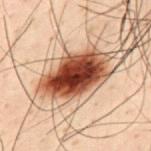workup: total-body-photography surveillance lesion; no biopsy
image: total-body-photography crop, ~15 mm field of view
patient: male, aged 28 to 32
tile lighting: cross-polarized
automated lesion analysis: a footprint of about 20 mm², a shape eccentricity near 0.8, and a symmetry-axis asymmetry near 0.2; a mean CIELAB color near L≈36 a*≈24 b*≈28, about 24 CIELAB-L* units darker than the surrounding skin, and a normalized border contrast of about 17.5; border irregularity of about 2.5 on a 0–10 scale and peripheral color asymmetry of about 2.5; a nevus-likeness score of about 100/100
lesion size: about 6.5 mm
location: the mid back